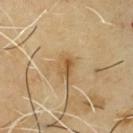No biopsy was performed on this lesion — it was imaged during a full skin examination and was not determined to be concerning. A lesion tile, about 15 mm wide, cut from a 3D total-body photograph. The subject is a male approximately 65 years of age. Located on the front of the torso.